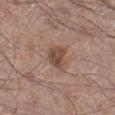{"biopsy_status": "not biopsied; imaged during a skin examination", "patient": {"sex": "male", "age_approx": 55}, "site": "right lower leg", "lesion_size": {"long_diameter_mm_approx": 3.0}, "image": {"source": "total-body photography crop", "field_of_view_mm": 15}, "lighting": "white-light"}